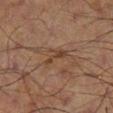biopsy status: imaged on a skin check; not biopsied.